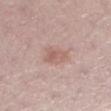follow-up — imaged on a skin check; not biopsied | diameter — ≈3 mm | automated lesion analysis — a mean CIELAB color near L≈59 a*≈20 b*≈24, a lesion–skin lightness drop of about 8, and a normalized lesion–skin contrast near 5.5; border irregularity of about 3 on a 0–10 scale, a within-lesion color-variation index near 3.5/10, and peripheral color asymmetry of about 1; lesion-presence confidence of about 100/100 | body site — the left lower leg | tile lighting — white-light | patient — female, about 50 years old | imaging modality — total-body-photography crop, ~15 mm field of view.